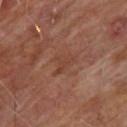The lesion was photographed on a routine skin check and not biopsied; there is no pathology result. Captured under cross-polarized illumination. On the upper back. Approximately 3.5 mm at its widest. The total-body-photography lesion software estimated a footprint of about 5.5 mm², an outline eccentricity of about 0.75 (0 = round, 1 = elongated), and a symmetry-axis asymmetry near 0.5. The software also gave a lesion color around L≈40 a*≈22 b*≈27 in CIELAB, about 5 CIELAB-L* units darker than the surrounding skin, and a normalized lesion–skin contrast near 5. The software also gave border irregularity of about 5 on a 0–10 scale, a color-variation rating of about 1.5/10, and peripheral color asymmetry of about 0.5. The software also gave a lesion-detection confidence of about 95/100. A region of skin cropped from a whole-body photographic capture, roughly 15 mm wide. The patient is a male roughly 65 years of age.